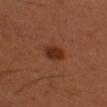The lesion-visualizer software estimated border irregularity of about 2 on a 0–10 scale, a color-variation rating of about 2.5/10, and a peripheral color-asymmetry measure near 1. Longest diameter approximately 3 mm. The tile uses cross-polarized illumination. A male patient in their 50s. A 15 mm close-up tile from a total-body photography series done for melanoma screening. The lesion is on the left thigh.